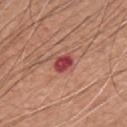Captured during whole-body skin photography for melanoma surveillance; the lesion was not biopsied. Cropped from a whole-body photographic skin survey; the tile spans about 15 mm. From the chest. A male patient, about 60 years old.